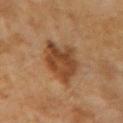  biopsy_status: not biopsied; imaged during a skin examination
  lighting: cross-polarized
  site: left upper arm
  lesion_size:
    long_diameter_mm_approx: 5.5
  patient:
    sex: female
    age_approx: 60
  image:
    source: total-body photography crop
    field_of_view_mm: 15